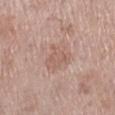Imaged during a routine full-body skin examination; the lesion was not biopsied and no histopathology is available. A female patient, roughly 70 years of age. From the right lower leg. The lesion's longest dimension is about 3 mm. The lesion-visualizer software estimated an eccentricity of roughly 0.3 and a shape-asymmetry score of about 0.4 (0 = symmetric). The software also gave about 7 CIELAB-L* units darker than the surrounding skin and a normalized border contrast of about 5. The software also gave internal color variation of about 2 on a 0–10 scale and peripheral color asymmetry of about 0.5. A close-up tile cropped from a whole-body skin photograph, about 15 mm across.